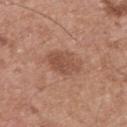Q: How was this image acquired?
A: ~15 mm crop, total-body skin-cancer survey
Q: What is the anatomic site?
A: the upper back
Q: How was the tile lit?
A: white-light illumination
Q: How large is the lesion?
A: ≈4 mm
Q: Who is the patient?
A: male, aged around 55